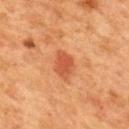Notes:
- workup: total-body-photography surveillance lesion; no biopsy
- image: total-body-photography crop, ~15 mm field of view
- automated lesion analysis: a lesion area of about 5.5 mm², an eccentricity of roughly 0.7, and a symmetry-axis asymmetry near 0.25; border irregularity of about 2.5 on a 0–10 scale, internal color variation of about 2 on a 0–10 scale, and radial color variation of about 1
- lesion size: ≈3.5 mm
- body site: the mid back
- patient: female, about 40 years old